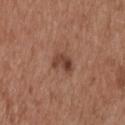Captured under white-light illumination. The lesion is located on the upper back. The total-body-photography lesion software estimated an area of roughly 5 mm². It also reported a within-lesion color-variation index near 5.5/10. And it measured an automated nevus-likeness rating near 75 out of 100 and a detector confidence of about 100 out of 100 that the crop contains a lesion. This image is a 15 mm lesion crop taken from a total-body photograph. The subject is a male aged approximately 55.